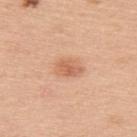{"biopsy_status": "not biopsied; imaged during a skin examination", "automated_metrics": {"area_mm2_approx": 5.0, "border_irregularity_0_10": 2.0, "peripheral_color_asymmetry": 1.0, "nevus_likeness_0_100": 90, "lesion_detection_confidence_0_100": 100}, "lesion_size": {"long_diameter_mm_approx": 3.0}, "patient": {"sex": "male", "age_approx": 50}, "site": "upper back", "image": {"source": "total-body photography crop", "field_of_view_mm": 15}}